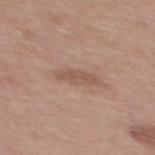  biopsy_status: not biopsied; imaged during a skin examination
  image:
    source: total-body photography crop
    field_of_view_mm: 15
  patient:
    sex: male
    age_approx: 30
  automated_metrics:
    eccentricity: 0.9
    shape_asymmetry: 0.2
    cielab_L: 54
    cielab_a: 19
    cielab_b: 27
    vs_skin_darker_L: 8.0
    vs_skin_contrast_norm: 6.0
  lesion_size:
    long_diameter_mm_approx: 4.0
  site: back